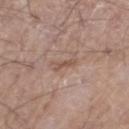  image:
    source: total-body photography crop
    field_of_view_mm: 15
  lighting: white-light
  site: left thigh
  lesion_size:
    long_diameter_mm_approx: 3.0
  patient:
    sex: male
    age_approx: 60